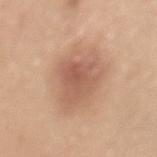Part of a total-body skin-imaging series; this lesion was reviewed on a skin check and was not flagged for biopsy.
The lesion's longest dimension is about 6 mm.
A male subject roughly 50 years of age.
A region of skin cropped from a whole-body photographic capture, roughly 15 mm wide.
Located on the back.
This is a white-light tile.